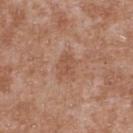Q: Is there a histopathology result?
A: no biopsy performed (imaged during a skin exam)
Q: Lesion location?
A: the upper back
Q: What kind of image is this?
A: ~15 mm crop, total-body skin-cancer survey
Q: Patient demographics?
A: male, aged around 45